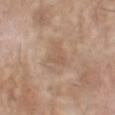No biopsy was performed on this lesion — it was imaged during a full skin examination and was not determined to be concerning.
The patient is a male approximately 70 years of age.
The lesion is on the head or neck.
Captured under white-light illumination.
A lesion tile, about 15 mm wide, cut from a 3D total-body photograph.